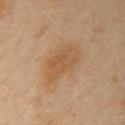Part of a total-body skin-imaging series; this lesion was reviewed on a skin check and was not flagged for biopsy.
The tile uses cross-polarized illumination.
A lesion tile, about 15 mm wide, cut from a 3D total-body photograph.
The subject is a female in their mid-40s.
The total-body-photography lesion software estimated a lesion area of about 14 mm², an eccentricity of roughly 0.8, and a shape-asymmetry score of about 0.2 (0 = symmetric). And it measured a mean CIELAB color near L≈46 a*≈16 b*≈32, roughly 6 lightness units darker than nearby skin, and a lesion-to-skin contrast of about 6 (normalized; higher = more distinct). And it measured a within-lesion color-variation index near 3/10 and a peripheral color-asymmetry measure near 1.
Measured at roughly 5.5 mm in maximum diameter.
On the arm.